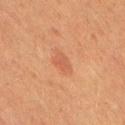Recorded during total-body skin imaging; not selected for excision or biopsy.
A 15 mm crop from a total-body photograph taken for skin-cancer surveillance.
The lesion is on the right thigh.
A female subject aged 48 to 52.